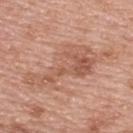Case summary:
* workup · no biopsy performed (imaged during a skin exam)
* image source · ~15 mm crop, total-body skin-cancer survey
* subject · female, aged 58 to 62
* site · the upper back
* lighting · white-light illumination
* automated metrics · a lesion area of about 18 mm² and a symmetry-axis asymmetry near 0.65; roughly 9 lightness units darker than nearby skin and a normalized lesion–skin contrast near 6; an automated nevus-likeness rating near 0 out of 100
* lesion size · about 8.5 mm